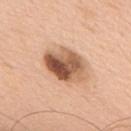Clinical impression: Imaged during a routine full-body skin examination; the lesion was not biopsied and no histopathology is available. Acquisition and patient details: A male subject, roughly 60 years of age. Cropped from a total-body skin-imaging series; the visible field is about 15 mm. This is a white-light tile. The lesion is located on the back. The recorded lesion diameter is about 5.5 mm.